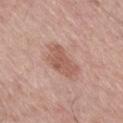Clinical summary: A close-up tile cropped from a whole-body skin photograph, about 15 mm across. A male subject, in their mid- to late 70s. The tile uses white-light illumination. The lesion is located on the leg.